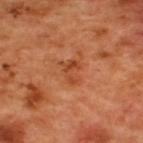This lesion was catalogued during total-body skin photography and was not selected for biopsy.